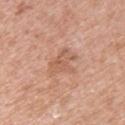Clinical impression:
Recorded during total-body skin imaging; not selected for excision or biopsy.
Context:
The recorded lesion diameter is about 4.5 mm. The lesion-visualizer software estimated a lesion area of about 8.5 mm², an outline eccentricity of about 0.8 (0 = round, 1 = elongated), and a symmetry-axis asymmetry near 0.4. It also reported a classifier nevus-likeness of about 0/100 and a detector confidence of about 100 out of 100 that the crop contains a lesion. The lesion is located on the upper back. This is a white-light tile. A roughly 15 mm field-of-view crop from a total-body skin photograph. A female subject, aged approximately 40.